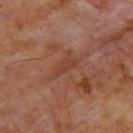Clinical impression:
No biopsy was performed on this lesion — it was imaged during a full skin examination and was not determined to be concerning.
Acquisition and patient details:
Captured under cross-polarized illumination. The lesion is on the upper back. Automated image analysis of the tile measured a border-irregularity index near 4.5/10 and radial color variation of about 0. The software also gave a nevus-likeness score of about 0/100. A male patient aged around 60. A region of skin cropped from a whole-body photographic capture, roughly 15 mm wide.